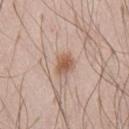workup=catalogued during a skin exam; not biopsied | lesion size=about 2.5 mm | image-analysis metrics=an average lesion color of about L≈57 a*≈19 b*≈29 (CIELAB) and about 11 CIELAB-L* units darker than the surrounding skin; a color-variation rating of about 4.5/10 and radial color variation of about 1.5 | acquisition=15 mm crop, total-body photography | anatomic site=the chest | subject=male, aged approximately 45 | illumination=white-light illumination.